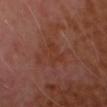Clinical impression: The lesion was tiled from a total-body skin photograph and was not biopsied. Acquisition and patient details: A male patient, in their mid-50s. The lesion is on the head or neck. This image is a 15 mm lesion crop taken from a total-body photograph.